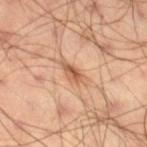The lesion was tiled from a total-body skin photograph and was not biopsied.
Approximately 4 mm at its widest.
Located on the right thigh.
The subject is a male aged approximately 55.
Imaged with cross-polarized lighting.
This image is a 15 mm lesion crop taken from a total-body photograph.